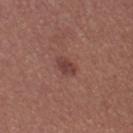{
  "biopsy_status": "not biopsied; imaged during a skin examination",
  "automated_metrics": {
    "area_mm2_approx": 3.5,
    "shape_asymmetry": 0.25,
    "border_irregularity_0_10": 2.5,
    "color_variation_0_10": 2.0,
    "peripheral_color_asymmetry": 1.0,
    "nevus_likeness_0_100": 80,
    "lesion_detection_confidence_0_100": 100
  },
  "site": "left thigh",
  "lesion_size": {
    "long_diameter_mm_approx": 2.5
  },
  "patient": {
    "sex": "female",
    "age_approx": 25
  },
  "lighting": "white-light",
  "image": {
    "source": "total-body photography crop",
    "field_of_view_mm": 15
  }
}